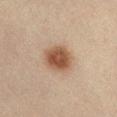Recorded during total-body skin imaging; not selected for excision or biopsy. A region of skin cropped from a whole-body photographic capture, roughly 15 mm wide. Located on the front of the torso. The lesion's longest dimension is about 4 mm. A female subject in their 60s.